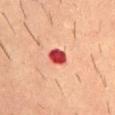biopsy status: imaged on a skin check; not biopsied
image: 15 mm crop, total-body photography
image-analysis metrics: an area of roughly 4.5 mm², an eccentricity of roughly 0.6, and a symmetry-axis asymmetry near 0.2; a peripheral color-asymmetry measure near 1; a classifier nevus-likeness of about 0/100
body site: the lower back
patient: male, aged 53 to 57
lesion diameter: ~2.5 mm (longest diameter)